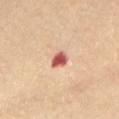This lesion was catalogued during total-body skin photography and was not selected for biopsy.
The subject is a female aged approximately 70.
The tile uses cross-polarized illumination.
On the abdomen.
About 2.5 mm across.
Automated image analysis of the tile measured a border-irregularity index near 2/10 and peripheral color asymmetry of about 1. It also reported an automated nevus-likeness rating near 0 out of 100 and a lesion-detection confidence of about 100/100.
A region of skin cropped from a whole-body photographic capture, roughly 15 mm wide.